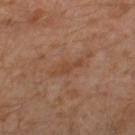Notes:
• notes · imaged on a skin check; not biopsied
• tile lighting · cross-polarized illumination
• site · the left thigh
• automated lesion analysis · two-axis asymmetry of about 0.4
• acquisition · total-body-photography crop, ~15 mm field of view
• patient · male, about 30 years old